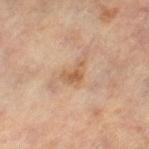Notes:
– workup: no biopsy performed (imaged during a skin exam)
– tile lighting: cross-polarized illumination
– diameter: ~2.5 mm (longest diameter)
– patient: female, approximately 65 years of age
– automated metrics: a border-irregularity rating of about 6/10, a within-lesion color-variation index near 2.5/10, and peripheral color asymmetry of about 1; a classifier nevus-likeness of about 5/100 and lesion-presence confidence of about 100/100
– imaging modality: total-body-photography crop, ~15 mm field of view
– location: the right leg